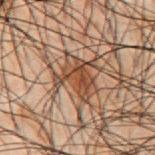- workup: catalogued during a skin exam; not biopsied
- patient: male, in their 50s
- lesion diameter: ≈5 mm
- illumination: cross-polarized illumination
- image: total-body-photography crop, ~15 mm field of view
- location: the back